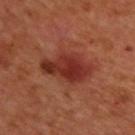Captured during whole-body skin photography for melanoma surveillance; the lesion was not biopsied.
Approximately 6 mm at its widest.
Captured under cross-polarized illumination.
The patient is a male aged 48 to 52.
A roughly 15 mm field-of-view crop from a total-body skin photograph.
On the upper back.